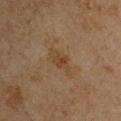Q: What are the patient's age and sex?
A: male, roughly 65 years of age
Q: What is the imaging modality?
A: ~15 mm tile from a whole-body skin photo
Q: Illumination type?
A: cross-polarized
Q: Lesion location?
A: the upper back
Q: How large is the lesion?
A: ~2.5 mm (longest diameter)
Q: Automated lesion metrics?
A: an area of roughly 3 mm² and a symmetry-axis asymmetry near 0.35; an average lesion color of about L≈32 a*≈14 b*≈27 (CIELAB) and a lesion–skin lightness drop of about 5; a within-lesion color-variation index near 1/10 and radial color variation of about 0.5